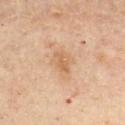Clinical impression: The lesion was photographed on a routine skin check and not biopsied; there is no pathology result. Image and clinical context: Imaged with cross-polarized lighting. A female subject approximately 45 years of age. A 15 mm close-up tile from a total-body photography series done for melanoma screening. The lesion-visualizer software estimated a lesion area of about 4 mm², a shape eccentricity near 0.85, and a shape-asymmetry score of about 0.25 (0 = symmetric). It also reported a mean CIELAB color near L≈60 a*≈20 b*≈35, a lesion–skin lightness drop of about 8, and a lesion-to-skin contrast of about 5.5 (normalized; higher = more distinct). It also reported a classifier nevus-likeness of about 0/100 and a detector confidence of about 100 out of 100 that the crop contains a lesion. On the chest.